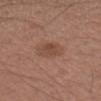image source — ~15 mm tile from a whole-body skin photo | lighting — white-light illumination | patient — male, in their 50s | body site — the arm | diameter — about 3.5 mm.